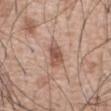Imaged during a routine full-body skin examination; the lesion was not biopsied and no histopathology is available.
From the front of the torso.
The tile uses white-light illumination.
Measured at roughly 3.5 mm in maximum diameter.
A close-up tile cropped from a whole-body skin photograph, about 15 mm across.
The patient is a male aged approximately 60.